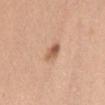Q: Is there a histopathology result?
A: imaged on a skin check; not biopsied
Q: What kind of image is this?
A: 15 mm crop, total-body photography
Q: Lesion size?
A: ≈2.5 mm
Q: Lesion location?
A: the front of the torso
Q: What lighting was used for the tile?
A: white-light illumination
Q: Who is the patient?
A: female, aged approximately 30